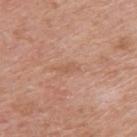<record>
<biopsy_status>not biopsied; imaged during a skin examination</biopsy_status>
<patient>
  <sex>male</sex>
  <age_approx>60</age_approx>
</patient>
<automated_metrics>
  <area_mm2_approx>2.5</area_mm2_approx>
  <eccentricity>0.9</eccentricity>
  <cielab_L>57</cielab_L>
  <cielab_a>22</cielab_a>
  <cielab_b>31</cielab_b>
  <vs_skin_darker_L>6.0</vs_skin_darker_L>
  <vs_skin_contrast_norm>4.5</vs_skin_contrast_norm>
  <border_irregularity_0_10>3.5</border_irregularity_0_10>
  <color_variation_0_10>0.0</color_variation_0_10>
  <peripheral_color_asymmetry>0.0</peripheral_color_asymmetry>
  <nevus_likeness_0_100>0</nevus_likeness_0_100>
  <lesion_detection_confidence_0_100>100</lesion_detection_confidence_0_100>
</automated_metrics>
<lighting>white-light</lighting>
<image>
  <source>total-body photography crop</source>
  <field_of_view_mm>15</field_of_view_mm>
</image>
<lesion_size>
  <long_diameter_mm_approx>2.5</long_diameter_mm_approx>
</lesion_size>
<site>right upper arm</site>
</record>